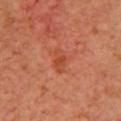The lesion was tiled from a total-body skin photograph and was not biopsied.
On the chest.
Automated image analysis of the tile measured an average lesion color of about L≈49 a*≈32 b*≈38 (CIELAB) and a lesion–skin lightness drop of about 7. The analysis additionally found a border-irregularity index near 3/10, internal color variation of about 2.5 on a 0–10 scale, and radial color variation of about 1.
Cropped from a total-body skin-imaging series; the visible field is about 15 mm.
Imaged with cross-polarized lighting.
The subject is a female aged 38–42.
About 3 mm across.